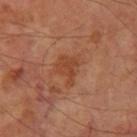This lesion was catalogued during total-body skin photography and was not selected for biopsy.
Automated tile analysis of the lesion measured a border-irregularity index near 5/10 and a peripheral color-asymmetry measure near 0.5. The analysis additionally found an automated nevus-likeness rating near 0 out of 100.
Imaged with cross-polarized lighting.
Approximately 4 mm at its widest.
Located on the right thigh.
The subject is a male aged approximately 70.
Cropped from a whole-body photographic skin survey; the tile spans about 15 mm.